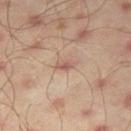No biopsy was performed on this lesion — it was imaged during a full skin examination and was not determined to be concerning.
The total-body-photography lesion software estimated an average lesion color of about L≈56 a*≈20 b*≈26 (CIELAB), roughly 9 lightness units darker than nearby skin, and a lesion-to-skin contrast of about 6 (normalized; higher = more distinct). It also reported border irregularity of about 3 on a 0–10 scale, a within-lesion color-variation index near 1/10, and radial color variation of about 0.5.
Imaged with cross-polarized lighting.
From the right thigh.
A male patient aged 43 to 47.
A region of skin cropped from a whole-body photographic capture, roughly 15 mm wide.